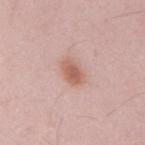Q: Was a biopsy performed?
A: no biopsy performed (imaged during a skin exam)
Q: Lesion location?
A: the mid back
Q: How was this image acquired?
A: ~15 mm crop, total-body skin-cancer survey
Q: What did automated image analysis measure?
A: an area of roughly 5.5 mm² and a shape-asymmetry score of about 0.15 (0 = symmetric); an average lesion color of about L≈60 a*≈23 b*≈27 (CIELAB); a within-lesion color-variation index near 2.5/10 and radial color variation of about 0.5; lesion-presence confidence of about 100/100
Q: What lighting was used for the tile?
A: white-light
Q: What is the lesion's diameter?
A: about 3 mm
Q: What are the patient's age and sex?
A: male, aged around 35